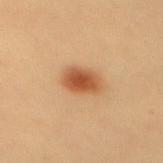biopsy status — total-body-photography surveillance lesion; no biopsy
acquisition — 15 mm crop, total-body photography
lighting — cross-polarized illumination
body site — the upper back
subject — female, aged around 30
lesion diameter — about 4 mm